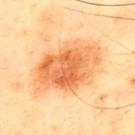workup: imaged on a skin check; not biopsied | site: the back | image-analysis metrics: an eccentricity of roughly 0.75 and two-axis asymmetry of about 0.2; an average lesion color of about L≈55 a*≈24 b*≈39 (CIELAB), roughly 12 lightness units darker than nearby skin, and a lesion-to-skin contrast of about 8 (normalized; higher = more distinct); a border-irregularity rating of about 3/10, internal color variation of about 6 on a 0–10 scale, and a peripheral color-asymmetry measure near 2 | lesion diameter: ~7.5 mm (longest diameter) | subject: male, in their mid- to late 40s | tile lighting: cross-polarized | acquisition: ~15 mm tile from a whole-body skin photo.